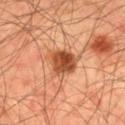Impression: Recorded during total-body skin imaging; not selected for excision or biopsy.